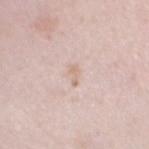{
  "patient": {
    "sex": "female",
    "age_approx": 30
  },
  "site": "chest",
  "image": {
    "source": "total-body photography crop",
    "field_of_view_mm": 15
  }
}